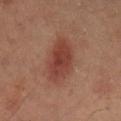follow-up = catalogued during a skin exam; not biopsied | illumination = cross-polarized | imaging modality = ~15 mm crop, total-body skin-cancer survey | patient = male, aged approximately 50 | automated metrics = about 8 CIELAB-L* units darker than the surrounding skin and a normalized border contrast of about 8 | anatomic site = the abdomen.